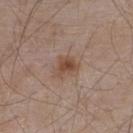{"patient": {"sex": "male", "age_approx": 55}, "image": {"source": "total-body photography crop", "field_of_view_mm": 15}, "site": "upper back", "lighting": "white-light"}